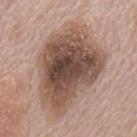Case summary:
– notes: catalogued during a skin exam; not biopsied
– body site: the mid back
– subject: male, aged around 70
– image source: ~15 mm crop, total-body skin-cancer survey